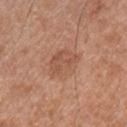Impression: Imaged during a routine full-body skin examination; the lesion was not biopsied and no histopathology is available. Context: The tile uses white-light illumination. A male patient, roughly 30 years of age. On the chest. Cropped from a total-body skin-imaging series; the visible field is about 15 mm. The lesion-visualizer software estimated a peripheral color-asymmetry measure near 1. The analysis additionally found an automated nevus-likeness rating near 10 out of 100. The lesion's longest dimension is about 4.5 mm.